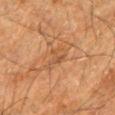The lesion is located on the right forearm. This image is a 15 mm lesion crop taken from a total-body photograph. A male patient, in their mid- to late 80s or older. The tile uses cross-polarized illumination.